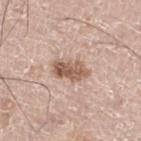| field | value |
|---|---|
| notes | imaged on a skin check; not biopsied |
| anatomic site | the leg |
| image source | 15 mm crop, total-body photography |
| lighting | white-light illumination |
| patient | male, aged around 70 |
| size | about 4 mm |